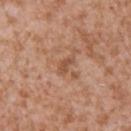biopsy status = no biopsy performed (imaged during a skin exam)
lighting = white-light
image = total-body-photography crop, ~15 mm field of view
lesion size = about 2.5 mm
automated lesion analysis = border irregularity of about 4 on a 0–10 scale, a within-lesion color-variation index near 0/10, and a peripheral color-asymmetry measure near 0; a nevus-likeness score of about 0/100 and a lesion-detection confidence of about 100/100
subject = male, aged around 45
site = the left upper arm